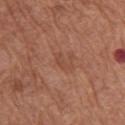follow-up — imaged on a skin check; not biopsied | subject — female, about 65 years old | image — 15 mm crop, total-body photography | lesion diameter — ≈3 mm | lighting — white-light | anatomic site — the left arm | TBP lesion metrics — a footprint of about 2.5 mm², an eccentricity of roughly 0.9, and two-axis asymmetry of about 0.55; a mean CIELAB color near L≈47 a*≈23 b*≈30 and roughly 6 lightness units darker than nearby skin; lesion-presence confidence of about 100/100.